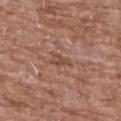<tbp_lesion>
<biopsy_status>not biopsied; imaged during a skin examination</biopsy_status>
<site>chest</site>
<lesion_size>
  <long_diameter_mm_approx>3.0</long_diameter_mm_approx>
</lesion_size>
<image>
  <source>total-body photography crop</source>
  <field_of_view_mm>15</field_of_view_mm>
</image>
<patient>
  <sex>male</sex>
  <age_approx>60</age_approx>
</patient>
</tbp_lesion>